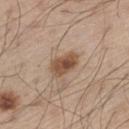No biopsy was performed on this lesion — it was imaged during a full skin examination and was not determined to be concerning. Located on the left thigh. Captured under white-light illumination. A male patient roughly 60 years of age. The recorded lesion diameter is about 4 mm. A region of skin cropped from a whole-body photographic capture, roughly 15 mm wide.